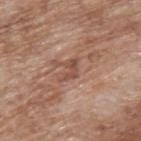Part of a total-body skin-imaging series; this lesion was reviewed on a skin check and was not flagged for biopsy. Captured under white-light illumination. On the back. The patient is a male aged around 70. Approximately 3 mm at its widest. The lesion-visualizer software estimated a lesion area of about 3 mm², a shape eccentricity near 0.85, and two-axis asymmetry of about 0.5. The software also gave an automated nevus-likeness rating near 0 out of 100. Cropped from a total-body skin-imaging series; the visible field is about 15 mm.